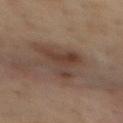Clinical impression:
Part of a total-body skin-imaging series; this lesion was reviewed on a skin check and was not flagged for biopsy.
Context:
The tile uses cross-polarized illumination. The lesion is located on the mid back. An algorithmic analysis of the crop reported an eccentricity of roughly 0.7 and a symmetry-axis asymmetry near 0.65. The analysis additionally found a lesion color around L≈38 a*≈16 b*≈23 in CIELAB, a lesion–skin lightness drop of about 8, and a normalized lesion–skin contrast near 7. And it measured a within-lesion color-variation index near 4.5/10 and a peripheral color-asymmetry measure near 1.5. The recorded lesion diameter is about 5.5 mm. A lesion tile, about 15 mm wide, cut from a 3D total-body photograph. The subject is a male in their mid-50s.